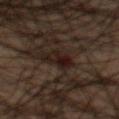Clinical impression:
Imaged during a routine full-body skin examination; the lesion was not biopsied and no histopathology is available.
Background:
From the mid back. The total-body-photography lesion software estimated a lesion color around L≈12 a*≈12 b*≈13 in CIELAB, roughly 6 lightness units darker than nearby skin, and a normalized lesion–skin contrast near 10. The software also gave a within-lesion color-variation index near 5/10. The software also gave a nevus-likeness score of about 0/100 and a lesion-detection confidence of about 90/100. A 15 mm close-up tile from a total-body photography series done for melanoma screening. The lesion's longest dimension is about 3.5 mm. A male patient roughly 50 years of age. This is a cross-polarized tile.